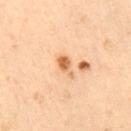From the right thigh. This is a cross-polarized tile. A male subject aged 63–67. A roughly 15 mm field-of-view crop from a total-body skin photograph. The total-body-photography lesion software estimated an average lesion color of about L≈68 a*≈24 b*≈42 (CIELAB) and about 13 CIELAB-L* units darker than the surrounding skin.